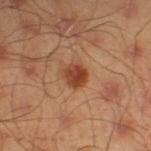{
  "biopsy_status": "not biopsied; imaged during a skin examination",
  "image": {
    "source": "total-body photography crop",
    "field_of_view_mm": 15
  },
  "site": "leg",
  "patient": {
    "sex": "male",
    "age_approx": 45
  }
}